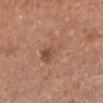Captured during whole-body skin photography for melanoma surveillance; the lesion was not biopsied. Measured at roughly 4 mm in maximum diameter. A lesion tile, about 15 mm wide, cut from a 3D total-body photograph. On the right lower leg. A male subject aged 53–57. Imaged with white-light lighting.